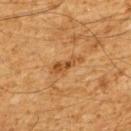Findings:
– workup — no biopsy performed (imaged during a skin exam)
– patient — male, roughly 60 years of age
– anatomic site — the upper back
– lesion size — about 4 mm
– lighting — cross-polarized
– imaging modality — total-body-photography crop, ~15 mm field of view